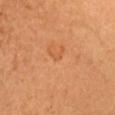Impression: Recorded during total-body skin imaging; not selected for excision or biopsy. Clinical summary: A region of skin cropped from a whole-body photographic capture, roughly 15 mm wide. The recorded lesion diameter is about 1 mm. The tile uses cross-polarized illumination. From the chest. The subject is a female about 45 years old.